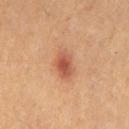Clinical impression: The lesion was photographed on a routine skin check and not biopsied; there is no pathology result. Image and clinical context: A female subject aged around 40. Cropped from a total-body skin-imaging series; the visible field is about 15 mm. The lesion's longest dimension is about 3 mm. The lesion is on the leg. The tile uses cross-polarized illumination. The total-body-photography lesion software estimated a mean CIELAB color near L≈55 a*≈27 b*≈33, a lesion–skin lightness drop of about 12, and a lesion-to-skin contrast of about 8 (normalized; higher = more distinct). The analysis additionally found a border-irregularity rating of about 2/10, internal color variation of about 4 on a 0–10 scale, and radial color variation of about 1. The software also gave an automated nevus-likeness rating near 95 out of 100 and lesion-presence confidence of about 100/100.